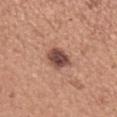Impression: No biopsy was performed on this lesion — it was imaged during a full skin examination and was not determined to be concerning. Acquisition and patient details: A roughly 15 mm field-of-view crop from a total-body skin photograph. About 3 mm across. From the left forearm. This is a white-light tile. The patient is a female aged 53–57.